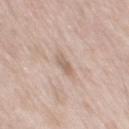Background:
The lesion is located on the mid back. Approximately 2.5 mm at its widest. This is a white-light tile. A male subject aged around 55. Cropped from a whole-body photographic skin survey; the tile spans about 15 mm.